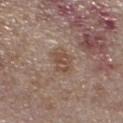The lesion was tiled from a total-body skin photograph and was not biopsied.
A female patient, approximately 65 years of age.
Captured under white-light illumination.
On the left lower leg.
A roughly 15 mm field-of-view crop from a total-body skin photograph.